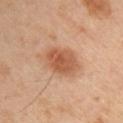Assessment: The lesion was tiled from a total-body skin photograph and was not biopsied. Background: Located on the left arm. About 5 mm across. A female subject approximately 40 years of age. Cropped from a total-body skin-imaging series; the visible field is about 15 mm.